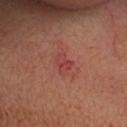Imaged during a routine full-body skin examination; the lesion was not biopsied and no histopathology is available. From the head or neck. A 15 mm crop from a total-body photograph taken for skin-cancer surveillance. The lesion's longest dimension is about 2.5 mm. Automated image analysis of the tile measured an area of roughly 3.5 mm² and a symmetry-axis asymmetry near 0.35. And it measured an average lesion color of about L≈44 a*≈32 b*≈26 (CIELAB), a lesion–skin lightness drop of about 6, and a lesion-to-skin contrast of about 5 (normalized; higher = more distinct). It also reported a border-irregularity index near 3/10, internal color variation of about 2.5 on a 0–10 scale, and a peripheral color-asymmetry measure near 1. Captured under cross-polarized illumination. A female subject, in their 50s.